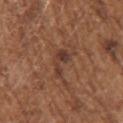| field | value |
|---|---|
| biopsy status | total-body-photography surveillance lesion; no biopsy |
| TBP lesion metrics | a lesion area of about 5.5 mm², an eccentricity of roughly 0.8, and two-axis asymmetry of about 0.5; a lesion–skin lightness drop of about 8; a detector confidence of about 100 out of 100 that the crop contains a lesion |
| subject | female, approximately 75 years of age |
| size | ~3.5 mm (longest diameter) |
| site | the left upper arm |
| image | total-body-photography crop, ~15 mm field of view |
| illumination | white-light illumination |